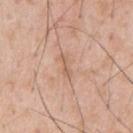notes = catalogued during a skin exam; not biopsied
subject = male, approximately 55 years of age
imaging modality = ~15 mm crop, total-body skin-cancer survey
anatomic site = the left upper arm